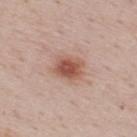The lesion was photographed on a routine skin check and not biopsied; there is no pathology result.
From the upper back.
A male patient in their 50s.
A close-up tile cropped from a whole-body skin photograph, about 15 mm across.